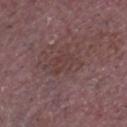Findings:
- biopsy status — catalogued during a skin exam; not biopsied
- patient — male, about 65 years old
- body site — the head or neck
- illumination — white-light illumination
- image source — ~15 mm crop, total-body skin-cancer survey
- size — ~4.5 mm (longest diameter)
- TBP lesion metrics — a footprint of about 9 mm² and a symmetry-axis asymmetry near 0.4; a classifier nevus-likeness of about 0/100 and lesion-presence confidence of about 85/100